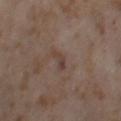lesion_size:
  long_diameter_mm_approx: 3.0
site: left thigh
patient:
  sex: female
  age_approx: 55
lighting: cross-polarized
image:
  source: total-body photography crop
  field_of_view_mm: 15
automated_metrics:
  area_mm2_approx: 2.5
  eccentricity: 0.95
  shape_asymmetry: 0.5
  cielab_L: 40
  cielab_a: 16
  cielab_b: 21
  vs_skin_darker_L: 7.0
  vs_skin_contrast_norm: 6.0
  nevus_likeness_0_100: 0
  lesion_detection_confidence_0_100: 100